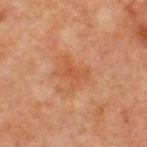Captured during whole-body skin photography for melanoma surveillance; the lesion was not biopsied. This is a cross-polarized tile. A male patient approximately 65 years of age. An algorithmic analysis of the crop reported an area of roughly 5 mm², an outline eccentricity of about 0.75 (0 = round, 1 = elongated), and a shape-asymmetry score of about 0.35 (0 = symmetric). It also reported border irregularity of about 4.5 on a 0–10 scale and a within-lesion color-variation index near 1.5/10. Located on the front of the torso. A lesion tile, about 15 mm wide, cut from a 3D total-body photograph.